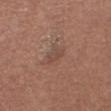* notes: total-body-photography surveillance lesion; no biopsy
* location: the right thigh
* patient: female, about 55 years old
* acquisition: total-body-photography crop, ~15 mm field of view